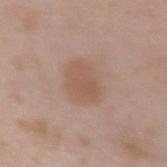This lesion was catalogued during total-body skin photography and was not selected for biopsy.
The lesion-visualizer software estimated an outline eccentricity of about 0.65 (0 = round, 1 = elongated) and a symmetry-axis asymmetry near 0.15. The software also gave an automated nevus-likeness rating near 25 out of 100 and a lesion-detection confidence of about 100/100.
A female subject about 50 years old.
The tile uses white-light illumination.
Approximately 4.5 mm at its widest.
From the left forearm.
A lesion tile, about 15 mm wide, cut from a 3D total-body photograph.